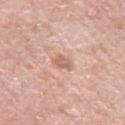Imaged during a routine full-body skin examination; the lesion was not biopsied and no histopathology is available. The recorded lesion diameter is about 2.5 mm. Automated tile analysis of the lesion measured a lesion area of about 4 mm² and a shape eccentricity near 0.8. It also reported a mean CIELAB color near L≈63 a*≈23 b*≈30, roughly 10 lightness units darker than nearby skin, and a normalized lesion–skin contrast near 6.5. The patient is a female about 65 years old. Imaged with white-light lighting. Located on the left upper arm. A roughly 15 mm field-of-view crop from a total-body skin photograph.